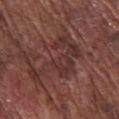{"biopsy_status": "not biopsied; imaged during a skin examination", "lesion_size": {"long_diameter_mm_approx": 7.0}, "image": {"source": "total-body photography crop", "field_of_view_mm": 15}, "site": "chest", "patient": {"sex": "male", "age_approx": 75}}